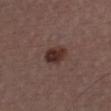Assessment: This lesion was catalogued during total-body skin photography and was not selected for biopsy. Background: Imaged with white-light lighting. A male subject, aged approximately 40. The lesion is on the front of the torso. Approximately 3.5 mm at its widest. A lesion tile, about 15 mm wide, cut from a 3D total-body photograph.